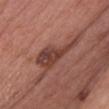Clinical impression: Imaged during a routine full-body skin examination; the lesion was not biopsied and no histopathology is available. Clinical summary: The patient is a female in their 60s. Located on the chest. A 15 mm close-up tile from a total-body photography series done for melanoma screening. Captured under white-light illumination.